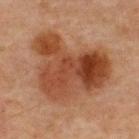Case summary:
* workup: catalogued during a skin exam; not biopsied
* body site: the upper back
* acquisition: total-body-photography crop, ~15 mm field of view
* TBP lesion metrics: about 10 CIELAB-L* units darker than the surrounding skin and a normalized lesion–skin contrast near 9; an automated nevus-likeness rating near 90 out of 100 and lesion-presence confidence of about 100/100
* lesion diameter: ≈9.5 mm
* lighting: cross-polarized illumination
* patient: male, approximately 60 years of age